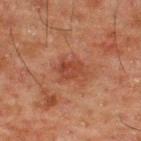Captured during whole-body skin photography for melanoma surveillance; the lesion was not biopsied. Located on the back. The tile uses cross-polarized illumination. The total-body-photography lesion software estimated an area of roughly 6 mm² and an outline eccentricity of about 0.65 (0 = round, 1 = elongated). The analysis additionally found a lesion color around L≈34 a*≈22 b*≈26 in CIELAB, a lesion–skin lightness drop of about 7, and a normalized border contrast of about 6.5. The analysis additionally found a border-irregularity rating of about 3/10. The software also gave a nevus-likeness score of about 0/100 and a detector confidence of about 100 out of 100 that the crop contains a lesion. A male subject about 50 years old. This image is a 15 mm lesion crop taken from a total-body photograph.